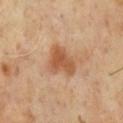The lesion was photographed on a routine skin check and not biopsied; there is no pathology result. About 4 mm across. On the chest. The patient is a male roughly 70 years of age. A lesion tile, about 15 mm wide, cut from a 3D total-body photograph. Imaged with cross-polarized lighting.